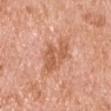follow-up = no biopsy performed (imaged during a skin exam)
anatomic site = the arm
imaging modality = 15 mm crop, total-body photography
lesion diameter = about 5.5 mm
tile lighting = white-light
patient = male, roughly 70 years of age